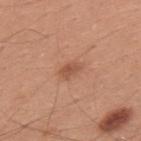Captured during whole-body skin photography for melanoma surveillance; the lesion was not biopsied. A male patient, aged 28 to 32. Longest diameter approximately 2.5 mm. Located on the upper back. A roughly 15 mm field-of-view crop from a total-body skin photograph.